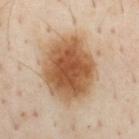follow-up: catalogued during a skin exam; not biopsied
patient: male, aged 53–57
illumination: cross-polarized illumination
image: ~15 mm tile from a whole-body skin photo
location: the chest